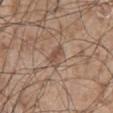Q: Was this lesion biopsied?
A: imaged on a skin check; not biopsied
Q: What lighting was used for the tile?
A: white-light illumination
Q: Who is the patient?
A: male, about 55 years old
Q: What is the lesion's diameter?
A: ≈2.5 mm
Q: What is the imaging modality?
A: ~15 mm tile from a whole-body skin photo
Q: What is the anatomic site?
A: the abdomen